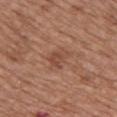Q: What lighting was used for the tile?
A: white-light illumination
Q: What kind of image is this?
A: ~15 mm tile from a whole-body skin photo
Q: Lesion location?
A: the chest
Q: How large is the lesion?
A: ~2.5 mm (longest diameter)
Q: Who is the patient?
A: female, in their mid- to late 70s